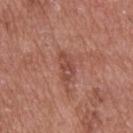notes: imaged on a skin check; not biopsied.